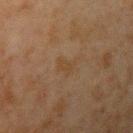Part of a total-body skin-imaging series; this lesion was reviewed on a skin check and was not flagged for biopsy.
A male patient, about 45 years old.
The lesion is on the right upper arm.
A roughly 15 mm field-of-view crop from a total-body skin photograph.
The lesion-visualizer software estimated a mean CIELAB color near L≈35 a*≈14 b*≈27 and a lesion-to-skin contrast of about 5 (normalized; higher = more distinct). It also reported a classifier nevus-likeness of about 0/100 and a lesion-detection confidence of about 100/100.
About 2.5 mm across.